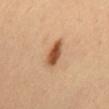This lesion was catalogued during total-body skin photography and was not selected for biopsy. On the mid back. A male patient, roughly 40 years of age. This is a cross-polarized tile. This image is a 15 mm lesion crop taken from a total-body photograph.